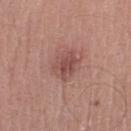biopsy status: no biopsy performed (imaged during a skin exam)
acquisition: 15 mm crop, total-body photography
anatomic site: the left lower leg
subject: male, aged 48 to 52
diameter: about 3.5 mm
illumination: white-light illumination
automated metrics: a lesion color around L≈49 a*≈23 b*≈23 in CIELAB and a lesion–skin lightness drop of about 9; an automated nevus-likeness rating near 45 out of 100